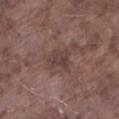  biopsy_status: not biopsied; imaged during a skin examination
  automated_metrics:
    area_mm2_approx: 6.5
    eccentricity: 0.5
    cielab_L: 40
    cielab_a: 17
    cielab_b: 19
    vs_skin_darker_L: 7.0
    border_irregularity_0_10: 3.0
    color_variation_0_10: 2.5
    peripheral_color_asymmetry: 1.0
    lesion_detection_confidence_0_100: 100
  site: left lower leg
  lesion_size:
    long_diameter_mm_approx: 3.0
  patient:
    sex: male
    age_approx: 75
  lighting: white-light
  image:
    source: total-body photography crop
    field_of_view_mm: 15